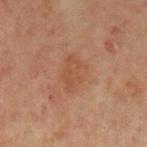This lesion was catalogued during total-body skin photography and was not selected for biopsy. The lesion's longest dimension is about 4 mm. A male patient aged approximately 45. The lesion is on the left upper arm. The tile uses cross-polarized illumination. A 15 mm close-up tile from a total-body photography series done for melanoma screening.